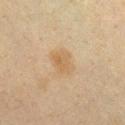biopsy status: total-body-photography surveillance lesion; no biopsy | automated lesion analysis: an eccentricity of roughly 0.65 and a symmetry-axis asymmetry near 0.2; border irregularity of about 2 on a 0–10 scale and radial color variation of about 1; an automated nevus-likeness rating near 20 out of 100 and a detector confidence of about 100 out of 100 that the crop contains a lesion | diameter: about 3 mm | illumination: cross-polarized | imaging modality: total-body-photography crop, ~15 mm field of view | patient: female, aged 38 to 42 | location: the chest.